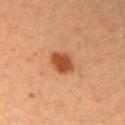Impression: Recorded during total-body skin imaging; not selected for excision or biopsy. Image and clinical context: This image is a 15 mm lesion crop taken from a total-body photograph. A female subject approximately 40 years of age. On the right upper arm.